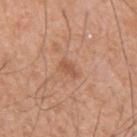Q: Was a biopsy performed?
A: catalogued during a skin exam; not biopsied
Q: What are the patient's age and sex?
A: male, about 55 years old
Q: Lesion location?
A: the left upper arm
Q: What is the imaging modality?
A: ~15 mm crop, total-body skin-cancer survey
Q: What did automated image analysis measure?
A: an average lesion color of about L≈55 a*≈23 b*≈33 (CIELAB); a border-irregularity rating of about 3.5/10, internal color variation of about 1 on a 0–10 scale, and a peripheral color-asymmetry measure near 0.5
Q: How large is the lesion?
A: ≈2.5 mm